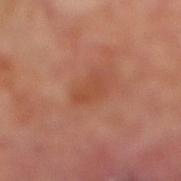follow-up: total-body-photography surveillance lesion; no biopsy
image source: ~15 mm crop, total-body skin-cancer survey
subject: male, about 70 years old
location: the right lower leg
diameter: ≈3.5 mm
image-analysis metrics: two-axis asymmetry of about 0.35; a mean CIELAB color near L≈47 a*≈26 b*≈32, roughly 6 lightness units darker than nearby skin, and a lesion-to-skin contrast of about 5 (normalized; higher = more distinct); border irregularity of about 4 on a 0–10 scale, a within-lesion color-variation index near 2/10, and peripheral color asymmetry of about 1; a classifier nevus-likeness of about 0/100
illumination: cross-polarized illumination